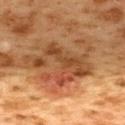  patient:
    sex: female
    age_approx: 40
  lesion_size:
    long_diameter_mm_approx: 8.5
  image:
    source: total-body photography crop
    field_of_view_mm: 15
  lighting: cross-polarized
  site: upper back